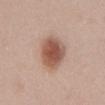The lesion was photographed on a routine skin check and not biopsied; there is no pathology result.
A male subject roughly 55 years of age.
The lesion is on the back.
Captured under white-light illumination.
A lesion tile, about 15 mm wide, cut from a 3D total-body photograph.
The total-body-photography lesion software estimated an average lesion color of about L≈54 a*≈21 b*≈27 (CIELAB), about 14 CIELAB-L* units darker than the surrounding skin, and a lesion-to-skin contrast of about 9.5 (normalized; higher = more distinct). It also reported a border-irregularity index near 2/10 and radial color variation of about 1.5. It also reported a nevus-likeness score of about 100/100 and lesion-presence confidence of about 100/100.